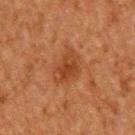follow-up — imaged on a skin check; not biopsied
body site — the upper back
subject — male, in their 50s
diameter — ≈4.5 mm
TBP lesion metrics — border irregularity of about 3 on a 0–10 scale, internal color variation of about 2.5 on a 0–10 scale, and peripheral color asymmetry of about 1; an automated nevus-likeness rating near 40 out of 100
image — ~15 mm crop, total-body skin-cancer survey
illumination — cross-polarized illumination